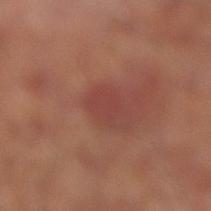| feature | finding |
|---|---|
| biopsy status | imaged on a skin check; not biopsied |
| lesion size | ~2.5 mm (longest diameter) |
| acquisition | 15 mm crop, total-body photography |
| anatomic site | the right lower leg |
| patient | male, about 65 years old |